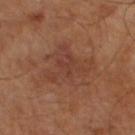Q: Was a biopsy performed?
A: imaged on a skin check; not biopsied